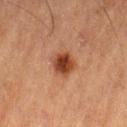biopsy_status: not biopsied; imaged during a skin examination
image:
  source: total-body photography crop
  field_of_view_mm: 15
lesion_size:
  long_diameter_mm_approx: 3.0
site: left thigh
patient:
  sex: male
  age_approx: 85+